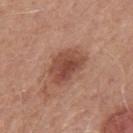The lesion was tiled from a total-body skin photograph and was not biopsied.
Imaged with white-light lighting.
Located on the mid back.
A male patient approximately 50 years of age.
A close-up tile cropped from a whole-body skin photograph, about 15 mm across.